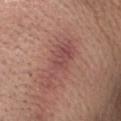Impression:
Imaged during a routine full-body skin examination; the lesion was not biopsied and no histopathology is available.
Context:
A male patient in their mid-50s. Measured at roughly 7 mm in maximum diameter. A 15 mm crop from a total-body photograph taken for skin-cancer surveillance. The total-body-photography lesion software estimated a footprint of about 12 mm² and a symmetry-axis asymmetry near 0.5. The analysis additionally found an average lesion color of about L≈49 a*≈24 b*≈23 (CIELAB), a lesion–skin lightness drop of about 8, and a lesion-to-skin contrast of about 6.5 (normalized; higher = more distinct). It also reported internal color variation of about 3 on a 0–10 scale and radial color variation of about 1. It also reported a classifier nevus-likeness of about 5/100 and a detector confidence of about 100 out of 100 that the crop contains a lesion. On the head or neck. Captured under white-light illumination.